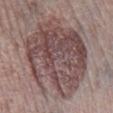Clinical impression: Part of a total-body skin-imaging series; this lesion was reviewed on a skin check and was not flagged for biopsy. Image and clinical context: The subject is a male in their mid-70s. From the right lower leg. A 15 mm crop from a total-body photograph taken for skin-cancer surveillance. Imaged with white-light lighting.